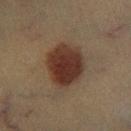Case summary:
– diameter · about 5.5 mm
– tile lighting · cross-polarized
– image-analysis metrics · a lesion area of about 19 mm², a shape eccentricity near 0.6, and a shape-asymmetry score of about 0.15 (0 = symmetric); a mean CIELAB color near L≈30 a*≈16 b*≈23, roughly 12 lightness units darker than nearby skin, and a normalized lesion–skin contrast near 11.5; a nevus-likeness score of about 100/100 and a detector confidence of about 100 out of 100 that the crop contains a lesion
– image · ~15 mm crop, total-body skin-cancer survey
– patient · male, aged 38–42
– location · the right lower leg